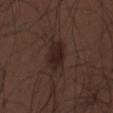Imaged during a routine full-body skin examination; the lesion was not biopsied and no histopathology is available. Longest diameter approximately 3.5 mm. Captured under white-light illumination. The subject is a male roughly 30 years of age. The lesion is on the chest. Cropped from a total-body skin-imaging series; the visible field is about 15 mm.